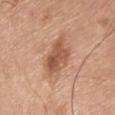patient:
  sex: male
  age_approx: 75
image:
  source: total-body photography crop
  field_of_view_mm: 15
automated_metrics:
  cielab_L: 54
  cielab_a: 23
  cielab_b: 32
  vs_skin_darker_L: 12.0
  color_variation_0_10: 4.0
  peripheral_color_asymmetry: 1.5
  nevus_likeness_0_100: 20
site: chest